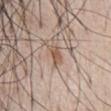Notes:
* follow-up — total-body-photography surveillance lesion; no biopsy
* image — total-body-photography crop, ~15 mm field of view
* tile lighting — white-light
* lesion size — ≈3 mm
* automated metrics — border irregularity of about 4.5 on a 0–10 scale and a color-variation rating of about 1/10; a classifier nevus-likeness of about 65/100 and lesion-presence confidence of about 95/100
* body site — the chest
* patient — male, in their 70s